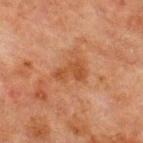Impression:
No biopsy was performed on this lesion — it was imaged during a full skin examination and was not determined to be concerning.
Context:
A male subject, aged approximately 65. This is a cross-polarized tile. On the chest. A lesion tile, about 15 mm wide, cut from a 3D total-body photograph. Longest diameter approximately 3.5 mm. The total-body-photography lesion software estimated a lesion area of about 5.5 mm², a shape eccentricity near 0.8, and two-axis asymmetry of about 0.5. The analysis additionally found a nevus-likeness score of about 0/100 and lesion-presence confidence of about 100/100.